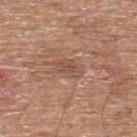Q: Was this lesion biopsied?
A: imaged on a skin check; not biopsied
Q: Who is the patient?
A: male, aged 63 to 67
Q: What is the anatomic site?
A: the upper back
Q: How was this image acquired?
A: ~15 mm crop, total-body skin-cancer survey
Q: Automated lesion metrics?
A: border irregularity of about 4 on a 0–10 scale, a within-lesion color-variation index near 2.5/10, and peripheral color asymmetry of about 1; a nevus-likeness score of about 0/100 and lesion-presence confidence of about 100/100